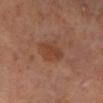Clinical impression: Part of a total-body skin-imaging series; this lesion was reviewed on a skin check and was not flagged for biopsy. Background: The subject is a male in their 60s. On the right lower leg. A roughly 15 mm field-of-view crop from a total-body skin photograph.